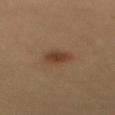| key | value |
|---|---|
| biopsy status | no biopsy performed (imaged during a skin exam) |
| imaging modality | ~15 mm tile from a whole-body skin photo |
| lighting | cross-polarized |
| diameter | ~3 mm (longest diameter) |
| site | the back |
| patient | female, aged around 35 |